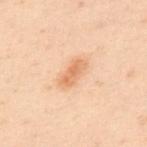Clinical impression:
This lesion was catalogued during total-body skin photography and was not selected for biopsy.
Image and clinical context:
A close-up tile cropped from a whole-body skin photograph, about 15 mm across. From the back. A male patient, about 50 years old. Approximately 3.5 mm at its widest. Captured under cross-polarized illumination.